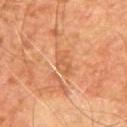follow-up = total-body-photography surveillance lesion; no biopsy
image = total-body-photography crop, ~15 mm field of view
site = the chest
lighting = cross-polarized
lesion size = ~3.5 mm (longest diameter)
subject = male, in their mid- to late 50s